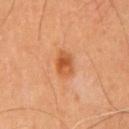Imaged during a routine full-body skin examination; the lesion was not biopsied and no histopathology is available.
A close-up tile cropped from a whole-body skin photograph, about 15 mm across.
An algorithmic analysis of the crop reported a mean CIELAB color near L≈53 a*≈27 b*≈40, about 10 CIELAB-L* units darker than the surrounding skin, and a normalized lesion–skin contrast near 8. The software also gave a border-irregularity index near 1.5/10 and internal color variation of about 5 on a 0–10 scale.
From the chest.
A male subject aged 58–62.
Measured at roughly 3.5 mm in maximum diameter.